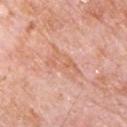{
  "biopsy_status": "not biopsied; imaged during a skin examination",
  "image": {
    "source": "total-body photography crop",
    "field_of_view_mm": 15
  },
  "site": "front of the torso",
  "lesion_size": {
    "long_diameter_mm_approx": 5.0
  },
  "automated_metrics": {
    "area_mm2_approx": 8.5,
    "eccentricity": 0.9,
    "shape_asymmetry": 0.35,
    "cielab_L": 65,
    "cielab_a": 24,
    "cielab_b": 33,
    "vs_skin_darker_L": 7.0,
    "border_irregularity_0_10": 4.5,
    "color_variation_0_10": 3.5,
    "peripheral_color_asymmetry": 1.0
  },
  "lighting": "white-light",
  "patient": {
    "sex": "male",
    "age_approx": 80
  }
}